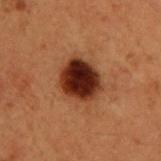Q: Was this lesion biopsied?
A: imaged on a skin check; not biopsied
Q: Lesion size?
A: ≈4 mm
Q: Automated lesion metrics?
A: an average lesion color of about L≈22 a*≈22 b*≈24 (CIELAB), a lesion–skin lightness drop of about 18, and a lesion-to-skin contrast of about 17.5 (normalized; higher = more distinct)
Q: How was this image acquired?
A: ~15 mm tile from a whole-body skin photo
Q: Who is the patient?
A: male, roughly 50 years of age
Q: Lesion location?
A: the back
Q: How was the tile lit?
A: cross-polarized